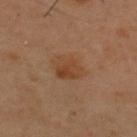{
  "biopsy_status": "not biopsied; imaged during a skin examination",
  "patient": {
    "sex": "male",
    "age_approx": 40
  },
  "site": "upper back",
  "lighting": "cross-polarized",
  "image": {
    "source": "total-body photography crop",
    "field_of_view_mm": 15
  },
  "automated_metrics": {
    "cielab_L": 42,
    "cielab_a": 20,
    "cielab_b": 32,
    "vs_skin_darker_L": 7.0,
    "vs_skin_contrast_norm": 6.5,
    "color_variation_0_10": 4.0,
    "nevus_likeness_0_100": 30,
    "lesion_detection_confidence_0_100": 100
  },
  "lesion_size": {
    "long_diameter_mm_approx": 3.5
  }
}